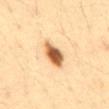Part of a total-body skin-imaging series; this lesion was reviewed on a skin check and was not flagged for biopsy. A close-up tile cropped from a whole-body skin photograph, about 15 mm across. Measured at roughly 4 mm in maximum diameter. Captured under cross-polarized illumination. The lesion is located on the abdomen. A male subject, aged around 35.